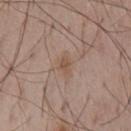  site: chest
  automated_metrics:
    cielab_L: 52
    cielab_a: 17
    cielab_b: 28
    vs_skin_darker_L: 7.0
    vs_skin_contrast_norm: 6.0
    border_irregularity_0_10: 3.0
    color_variation_0_10: 1.0
    peripheral_color_asymmetry: 0.5
  patient:
    sex: male
    age_approx: 65
  image:
    source: total-body photography crop
    field_of_view_mm: 15
  lesion_size:
    long_diameter_mm_approx: 2.5